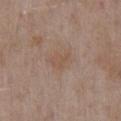This lesion was catalogued during total-body skin photography and was not selected for biopsy.
The patient is a male about 55 years old.
The lesion is located on the right upper arm.
An algorithmic analysis of the crop reported an average lesion color of about L≈52 a*≈16 b*≈27 (CIELAB), about 5 CIELAB-L* units darker than the surrounding skin, and a normalized border contrast of about 5. The software also gave a nevus-likeness score of about 0/100 and a lesion-detection confidence of about 100/100.
This image is a 15 mm lesion crop taken from a total-body photograph.
This is a white-light tile.
About 2.5 mm across.